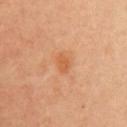Assessment: Part of a total-body skin-imaging series; this lesion was reviewed on a skin check and was not flagged for biopsy.